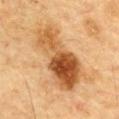Q: How was the tile lit?
A: cross-polarized
Q: Where on the body is the lesion?
A: the chest
Q: Patient demographics?
A: male, roughly 85 years of age or older
Q: What is the lesion's diameter?
A: about 9 mm
Q: What kind of image is this?
A: 15 mm crop, total-body photography